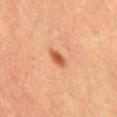Impression: Captured during whole-body skin photography for melanoma surveillance; the lesion was not biopsied. Clinical summary: The lesion's longest dimension is about 3 mm. This is a cross-polarized tile. Located on the abdomen. This image is a 15 mm lesion crop taken from a total-body photograph. A female patient.